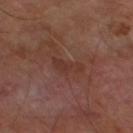Case summary:
- follow-up: catalogued during a skin exam; not biopsied
- subject: male, about 70 years old
- anatomic site: the right thigh
- imaging modality: total-body-photography crop, ~15 mm field of view